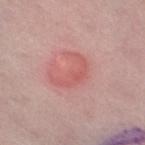A lesion tile, about 15 mm wide, cut from a 3D total-body photograph. Located on the right thigh. A female subject approximately 45 years of age. Biopsy histopathology demonstrated a dermatofibroma.